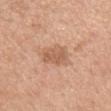No biopsy was performed on this lesion — it was imaged during a full skin examination and was not determined to be concerning. Cropped from a total-body skin-imaging series; the visible field is about 15 mm. A female subject aged 58 to 62. On the mid back.